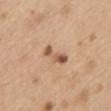A female subject aged approximately 45.
On the mid back.
A roughly 15 mm field-of-view crop from a total-body skin photograph.
This is a white-light tile.
The recorded lesion diameter is about 4 mm.